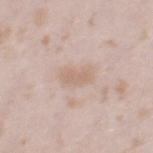Recorded during total-body skin imaging; not selected for excision or biopsy. Approximately 3.5 mm at its widest. The lesion is located on the leg. Imaged with white-light lighting. A 15 mm close-up tile from a total-body photography series done for melanoma screening. A female patient, roughly 25 years of age.